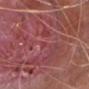Automated tile analysis of the lesion measured a lesion area of about 0.5 mm², an outline eccentricity of about 0.85 (0 = round, 1 = elongated), and a symmetry-axis asymmetry near 0.45. The software also gave a color-variation rating of about 0/10 and peripheral color asymmetry of about 0. A 15 mm close-up extracted from a 3D total-body photography capture. The patient is a male aged 63 to 67. The lesion is on the left lower leg. Histopathologically confirmed as a nodular basal cell carcinoma, classified as a malignancy.